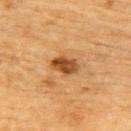The lesion was photographed on a routine skin check and not biopsied; there is no pathology result. On the upper back. The tile uses cross-polarized illumination. The subject is a male aged 83 or over. A lesion tile, about 15 mm wide, cut from a 3D total-body photograph. The total-body-photography lesion software estimated a footprint of about 6 mm², a shape eccentricity near 0.75, and a shape-asymmetry score of about 0.2 (0 = symmetric). The software also gave a mean CIELAB color near L≈43 a*≈22 b*≈37 and a lesion-to-skin contrast of about 10 (normalized; higher = more distinct). The lesion's longest dimension is about 3.5 mm.